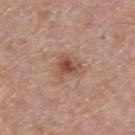Q: Was a biopsy performed?
A: imaged on a skin check; not biopsied
Q: Illumination type?
A: white-light illumination
Q: Lesion size?
A: about 3.5 mm
Q: What is the imaging modality?
A: 15 mm crop, total-body photography
Q: Patient demographics?
A: male, aged 53–57